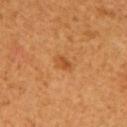{
  "biopsy_status": "not biopsied; imaged during a skin examination",
  "lighting": "cross-polarized",
  "image": {
    "source": "total-body photography crop",
    "field_of_view_mm": 15
  },
  "site": "back",
  "patient": {
    "sex": "male",
    "age_approx": 45
  },
  "lesion_size": {
    "long_diameter_mm_approx": 2.0
  }
}